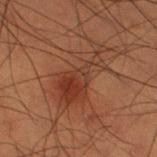| field | value |
|---|---|
| workup | catalogued during a skin exam; not biopsied |
| site | the right thigh |
| patient | male, in their 50s |
| image source | 15 mm crop, total-body photography |